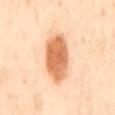Notes:
• biopsy status · catalogued during a skin exam; not biopsied
• image · ~15 mm crop, total-body skin-cancer survey
• image-analysis metrics · an area of roughly 17 mm², an eccentricity of roughly 0.85, and a shape-asymmetry score of about 0.15 (0 = symmetric)
• lighting · cross-polarized
• patient · female, aged 53 to 57
• body site · the back
• diameter · about 6.5 mm